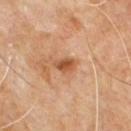automated lesion analysis: a mean CIELAB color near L≈51 a*≈23 b*≈35, about 11 CIELAB-L* units darker than the surrounding skin, and a normalized lesion–skin contrast near 8 | anatomic site: the upper back | acquisition: 15 mm crop, total-body photography | subject: male, aged 58 to 62 | illumination: cross-polarized illumination.